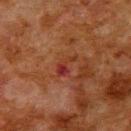| feature | finding |
|---|---|
| notes | no biopsy performed (imaged during a skin exam) |
| lighting | cross-polarized illumination |
| anatomic site | the upper back |
| patient | male, in their 80s |
| imaging modality | total-body-photography crop, ~15 mm field of view |
| diameter | about 3 mm |